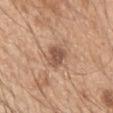No biopsy was performed on this lesion — it was imaged during a full skin examination and was not determined to be concerning. A 15 mm close-up extracted from a 3D total-body photography capture. Captured under white-light illumination. The subject is a male aged approximately 50. The lesion-visualizer software estimated border irregularity of about 2.5 on a 0–10 scale and radial color variation of about 1. The software also gave a nevus-likeness score of about 80/100 and lesion-presence confidence of about 100/100. From the left upper arm.